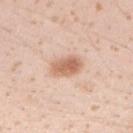Imaged during a routine full-body skin examination; the lesion was not biopsied and no histopathology is available.
This image is a 15 mm lesion crop taken from a total-body photograph.
The subject is a female in their 20s.
Imaged with white-light lighting.
From the right forearm.
Automated tile analysis of the lesion measured a lesion area of about 7.5 mm² and two-axis asymmetry of about 0.2. And it measured a mean CIELAB color near L≈65 a*≈21 b*≈32 and roughly 13 lightness units darker than nearby skin. The analysis additionally found a classifier nevus-likeness of about 95/100 and lesion-presence confidence of about 100/100.
The recorded lesion diameter is about 3.5 mm.